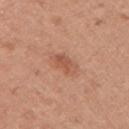biopsy_status: not biopsied; imaged during a skin examination
patient:
  sex: male
  age_approx: 50
image:
  source: total-body photography crop
  field_of_view_mm: 15
site: arm
lesion_size:
  long_diameter_mm_approx: 3.0